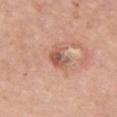biopsy status: total-body-photography surveillance lesion; no biopsy | lesion diameter: ~3 mm (longest diameter) | imaging modality: ~15 mm tile from a whole-body skin photo | patient: female, aged 63–67 | automated lesion analysis: a lesion area of about 5.5 mm², an eccentricity of roughly 0.7, and two-axis asymmetry of about 0.25; an average lesion color of about L≈56 a*≈23 b*≈29 (CIELAB), roughly 11 lightness units darker than nearby skin, and a lesion-to-skin contrast of about 7 (normalized; higher = more distinct); a border-irregularity rating of about 2/10, a within-lesion color-variation index near 6.5/10, and radial color variation of about 2 | location: the chest.